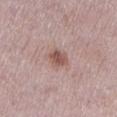<tbp_lesion>
  <biopsy_status>not biopsied; imaged during a skin examination</biopsy_status>
  <patient>
    <sex>female</sex>
    <age_approx>30</age_approx>
  </patient>
  <site>left lower leg</site>
  <image>
    <source>total-body photography crop</source>
    <field_of_view_mm>15</field_of_view_mm>
  </image>
  <lesion_size>
    <long_diameter_mm_approx>2.5</long_diameter_mm_approx>
  </lesion_size>
</tbp_lesion>